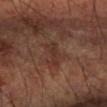Assessment: Part of a total-body skin-imaging series; this lesion was reviewed on a skin check and was not flagged for biopsy. Image and clinical context: Approximately 4 mm at its widest. A male subject, aged 58–62. The total-body-photography lesion software estimated an average lesion color of about L≈31 a*≈19 b*≈23 (CIELAB), a lesion–skin lightness drop of about 6, and a normalized border contrast of about 6. And it measured a color-variation rating of about 1.5/10. A 15 mm close-up tile from a total-body photography series done for melanoma screening. This is a cross-polarized tile. On the right forearm.